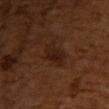The lesion was tiled from a total-body skin photograph and was not biopsied. Imaged with cross-polarized lighting. A region of skin cropped from a whole-body photographic capture, roughly 15 mm wide. A female subject approximately 55 years of age. The lesion is on the upper back.